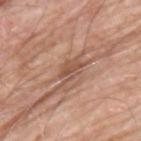Captured during whole-body skin photography for melanoma surveillance; the lesion was not biopsied. A male subject, aged around 65. The lesion-visualizer software estimated a mean CIELAB color near L≈53 a*≈21 b*≈29, a lesion–skin lightness drop of about 10, and a normalized lesion–skin contrast near 6.5. The software also gave internal color variation of about 3.5 on a 0–10 scale and a peripheral color-asymmetry measure near 1.5. Cropped from a whole-body photographic skin survey; the tile spans about 15 mm. Longest diameter approximately 4 mm. The lesion is on the back.